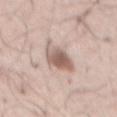Q: Was a biopsy performed?
A: catalogued during a skin exam; not biopsied
Q: What kind of image is this?
A: 15 mm crop, total-body photography
Q: Automated lesion metrics?
A: a border-irregularity rating of about 3/10 and radial color variation of about 1.5; a nevus-likeness score of about 95/100
Q: Who is the patient?
A: male, aged 58 to 62
Q: How large is the lesion?
A: ≈5 mm
Q: Illumination type?
A: white-light illumination
Q: What is the anatomic site?
A: the chest